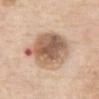Clinical impression: Part of a total-body skin-imaging series; this lesion was reviewed on a skin check and was not flagged for biopsy. Image and clinical context: A female subject, approximately 65 years of age. Captured under white-light illumination. Automated image analysis of the tile measured a lesion area of about 25 mm², an eccentricity of roughly 0.75, and a symmetry-axis asymmetry near 0.2. The analysis additionally found an average lesion color of about L≈59 a*≈18 b*≈30 (CIELAB), a lesion–skin lightness drop of about 16, and a normalized lesion–skin contrast near 10. On the abdomen. A close-up tile cropped from a whole-body skin photograph, about 15 mm across.